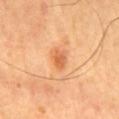  biopsy_status: not biopsied; imaged during a skin examination
  site: mid back
  image:
    source: total-body photography crop
    field_of_view_mm: 15
  patient:
    sex: male
    age_approx: 65